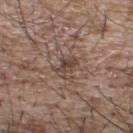Assessment: The lesion was photographed on a routine skin check and not biopsied; there is no pathology result. Background: A 15 mm crop from a total-body photograph taken for skin-cancer surveillance. Located on the back. The subject is a male aged 63 to 67.